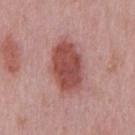biopsy status: no biopsy performed (imaged during a skin exam)
acquisition: ~15 mm tile from a whole-body skin photo
automated lesion analysis: a footprint of about 19 mm², an outline eccentricity of about 0.8 (0 = round, 1 = elongated), and a shape-asymmetry score of about 0.15 (0 = symmetric); a lesion–skin lightness drop of about 13 and a normalized border contrast of about 9.5; a classifier nevus-likeness of about 100/100 and lesion-presence confidence of about 100/100
body site: the chest
lighting: white-light illumination
diameter: ≈6 mm
patient: male, aged 48 to 52